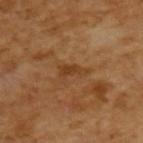Part of a total-body skin-imaging series; this lesion was reviewed on a skin check and was not flagged for biopsy.
A male patient, aged around 65.
The total-body-photography lesion software estimated an area of roughly 3.5 mm², an eccentricity of roughly 0.85, and a symmetry-axis asymmetry near 0.45. The software also gave an average lesion color of about L≈38 a*≈21 b*≈35 (CIELAB), roughly 7 lightness units darker than nearby skin, and a lesion-to-skin contrast of about 6.5 (normalized; higher = more distinct). The software also gave a classifier nevus-likeness of about 0/100 and a detector confidence of about 100 out of 100 that the crop contains a lesion.
A roughly 15 mm field-of-view crop from a total-body skin photograph.
This is a cross-polarized tile.
About 3 mm across.